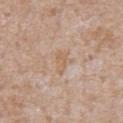Case summary:
– image — ~15 mm tile from a whole-body skin photo
– location — the chest
– patient — male, aged approximately 65